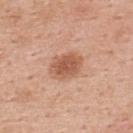Q: Is there a histopathology result?
A: imaged on a skin check; not biopsied
Q: What kind of image is this?
A: total-body-photography crop, ~15 mm field of view
Q: What is the lesion's diameter?
A: ≈4 mm
Q: Illumination type?
A: white-light illumination
Q: Lesion location?
A: the back
Q: Who is the patient?
A: female, aged 48–52
Q: What did automated image analysis measure?
A: a lesion area of about 9 mm², an outline eccentricity of about 0.65 (0 = round, 1 = elongated), and a symmetry-axis asymmetry near 0.15